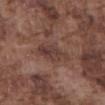Q: Was this lesion biopsied?
A: imaged on a skin check; not biopsied
Q: Where on the body is the lesion?
A: the abdomen
Q: What did automated image analysis measure?
A: a lesion area of about 8.5 mm² and an eccentricity of roughly 0.85; roughly 7 lightness units darker than nearby skin and a normalized border contrast of about 6.5; a border-irregularity index near 3.5/10, a color-variation rating of about 4/10, and radial color variation of about 1.5
Q: What kind of image is this?
A: total-body-photography crop, ~15 mm field of view
Q: Who is the patient?
A: male, about 75 years old
Q: Lesion size?
A: ≈4.5 mm